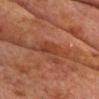Impression:
Recorded during total-body skin imaging; not selected for excision or biopsy.
Context:
About 2.5 mm across. A lesion tile, about 15 mm wide, cut from a 3D total-body photograph. Imaged with cross-polarized lighting. Located on the front of the torso. A male patient aged around 75.